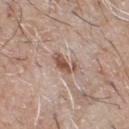| field | value |
|---|---|
| notes | catalogued during a skin exam; not biopsied |
| image | ~15 mm tile from a whole-body skin photo |
| image-analysis metrics | a mean CIELAB color near L≈53 a*≈19 b*≈26 and roughly 12 lightness units darker than nearby skin; a border-irregularity index near 4.5/10 and radial color variation of about 0.5; an automated nevus-likeness rating near 70 out of 100 and a detector confidence of about 100 out of 100 that the crop contains a lesion |
| illumination | white-light |
| size | about 3.5 mm |
| site | the chest |
| patient | male, aged approximately 65 |